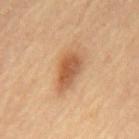Captured during whole-body skin photography for melanoma surveillance; the lesion was not biopsied. On the mid back. A roughly 15 mm field-of-view crop from a total-body skin photograph. Longest diameter approximately 5 mm. A male patient, aged 83 or over. The tile uses cross-polarized illumination.